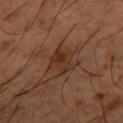The lesion was photographed on a routine skin check and not biopsied; there is no pathology result. A male patient about 65 years old. Imaged with cross-polarized lighting. A 15 mm close-up extracted from a 3D total-body photography capture. On the right thigh. The lesion-visualizer software estimated a mean CIELAB color near L≈31 a*≈20 b*≈27, about 8 CIELAB-L* units darker than the surrounding skin, and a normalized lesion–skin contrast near 7.5.